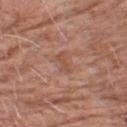Q: Patient demographics?
A: male, aged 78–82
Q: Illumination type?
A: white-light
Q: What kind of image is this?
A: 15 mm crop, total-body photography
Q: What did automated image analysis measure?
A: a border-irregularity rating of about 3.5/10 and radial color variation of about 0
Q: Lesion size?
A: ~2.5 mm (longest diameter)
Q: Where on the body is the lesion?
A: the chest